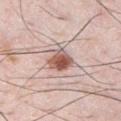Clinical impression:
No biopsy was performed on this lesion — it was imaged during a full skin examination and was not determined to be concerning.
Context:
Longest diameter approximately 3 mm. From the chest. The subject is a male in their mid- to late 30s. A region of skin cropped from a whole-body photographic capture, roughly 15 mm wide.